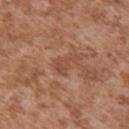A male patient, aged approximately 45.
Located on the right upper arm.
The lesion's longest dimension is about 3 mm.
This image is a 15 mm lesion crop taken from a total-body photograph.